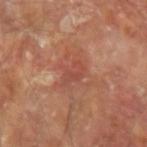The lesion was tiled from a total-body skin photograph and was not biopsied.
The lesion is on the left forearm.
Cropped from a whole-body photographic skin survey; the tile spans about 15 mm.
The subject is a male roughly 65 years of age.